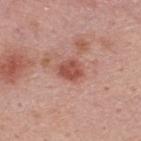A male patient aged 43–47. A lesion tile, about 15 mm wide, cut from a 3D total-body photograph. The total-body-photography lesion software estimated a lesion area of about 5.5 mm² and a shape-asymmetry score of about 0.2 (0 = symmetric). And it measured about 11 CIELAB-L* units darker than the surrounding skin and a normalized lesion–skin contrast near 8. And it measured a classifier nevus-likeness of about 30/100 and a lesion-detection confidence of about 100/100. The lesion is on the upper back.